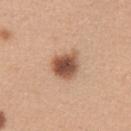{
  "image": {
    "source": "total-body photography crop",
    "field_of_view_mm": 15
  },
  "lighting": "white-light",
  "lesion_size": {
    "long_diameter_mm_approx": 4.0
  },
  "site": "right upper arm",
  "patient": {
    "sex": "female",
    "age_approx": 30
  }
}